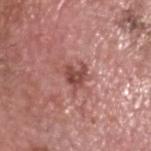The lesion was photographed on a routine skin check and not biopsied; there is no pathology result. Cropped from a total-body skin-imaging series; the visible field is about 15 mm. A male patient aged around 75. An algorithmic analysis of the crop reported an area of roughly 5.5 mm² and a symmetry-axis asymmetry near 0.4. It also reported a lesion color around L≈48 a*≈26 b*≈25 in CIELAB, roughly 11 lightness units darker than nearby skin, and a normalized lesion–skin contrast near 8. And it measured radial color variation of about 1. Imaged with white-light lighting. The lesion's longest dimension is about 3 mm. On the head or neck.